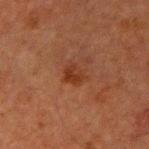The lesion was photographed on a routine skin check and not biopsied; there is no pathology result.